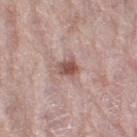Q: Was a biopsy performed?
A: catalogued during a skin exam; not biopsied
Q: What did automated image analysis measure?
A: an outline eccentricity of about 0.35 (0 = round, 1 = elongated) and a shape-asymmetry score of about 0.4 (0 = symmetric); a lesion color around L≈53 a*≈21 b*≈24 in CIELAB and a normalized border contrast of about 8.5; a border-irregularity index near 3.5/10, a color-variation rating of about 5/10, and radial color variation of about 1.5
Q: How was the tile lit?
A: white-light illumination
Q: What is the anatomic site?
A: the right thigh
Q: Who is the patient?
A: female, about 70 years old
Q: What kind of image is this?
A: total-body-photography crop, ~15 mm field of view
Q: How large is the lesion?
A: about 2.5 mm